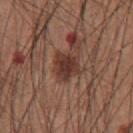Clinical impression: The lesion was photographed on a routine skin check and not biopsied; there is no pathology result. Image and clinical context: A male patient, aged 58 to 62. Cropped from a total-body skin-imaging series; the visible field is about 15 mm. This is a white-light tile. Located on the chest. Automated tile analysis of the lesion measured an area of roughly 7 mm², an eccentricity of roughly 0.5, and two-axis asymmetry of about 0.25. The software also gave an average lesion color of about L≈35 a*≈21 b*≈24 (CIELAB), about 11 CIELAB-L* units darker than the surrounding skin, and a normalized lesion–skin contrast near 9.5. And it measured border irregularity of about 2.5 on a 0–10 scale, a color-variation rating of about 3.5/10, and a peripheral color-asymmetry measure near 1. The software also gave an automated nevus-likeness rating near 10 out of 100 and a detector confidence of about 100 out of 100 that the crop contains a lesion.